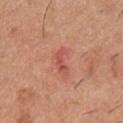No biopsy was performed on this lesion — it was imaged during a full skin examination and was not determined to be concerning. The patient is a male in their 40s. A 15 mm close-up extracted from a 3D total-body photography capture. The tile uses white-light illumination. The lesion is on the chest. Longest diameter approximately 3 mm.